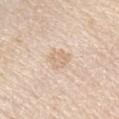tile lighting: white-light
automated metrics: a border-irregularity rating of about 2.5/10, a color-variation rating of about 2.5/10, and peripheral color asymmetry of about 1; a classifier nevus-likeness of about 0/100 and a detector confidence of about 95 out of 100 that the crop contains a lesion
image source: ~15 mm tile from a whole-body skin photo
body site: the left upper arm
patient: female, aged approximately 45
size: ~3 mm (longest diameter)